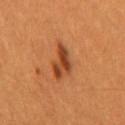Clinical impression: This lesion was catalogued during total-body skin photography and was not selected for biopsy. Background: A male patient, approximately 60 years of age. A close-up tile cropped from a whole-body skin photograph, about 15 mm across. From the mid back. An algorithmic analysis of the crop reported a border-irregularity index near 4/10 and peripheral color asymmetry of about 1.5. And it measured an automated nevus-likeness rating near 85 out of 100 and lesion-presence confidence of about 100/100.